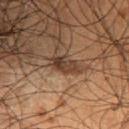Q: Is there a histopathology result?
A: catalogued during a skin exam; not biopsied